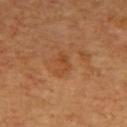Imaged during a routine full-body skin examination; the lesion was not biopsied and no histopathology is available. The tile uses cross-polarized illumination. Longest diameter approximately 3 mm. The patient is a male in their mid- to late 60s. Cropped from a total-body skin-imaging series; the visible field is about 15 mm. From the upper back.